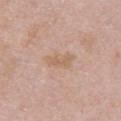Impression: Recorded during total-body skin imaging; not selected for excision or biopsy. Acquisition and patient details: This is a white-light tile. Located on the right upper arm. Measured at roughly 4 mm in maximum diameter. The subject is a female aged 28–32. A 15 mm close-up tile from a total-body photography series done for melanoma screening.